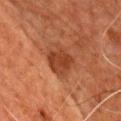About 4 mm across. The lesion is located on the chest. The total-body-photography lesion software estimated an area of roughly 10 mm², a shape eccentricity near 0.55, and a symmetry-axis asymmetry near 0.25. The software also gave an average lesion color of about L≈33 a*≈23 b*≈29 (CIELAB), about 8 CIELAB-L* units darker than the surrounding skin, and a normalized lesion–skin contrast near 7. The software also gave a border-irregularity rating of about 2.5/10 and a color-variation rating of about 2.5/10. Captured under cross-polarized illumination. A male subject, in their 80s. Cropped from a whole-body photographic skin survey; the tile spans about 15 mm.